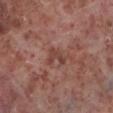notes = imaged on a skin check; not biopsied | size = about 3 mm | imaging modality = ~15 mm tile from a whole-body skin photo | subject = male, aged 53 to 57 | tile lighting = white-light | location = the left lower leg.